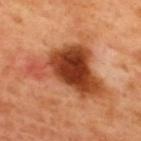Q: Is there a histopathology result?
A: catalogued during a skin exam; not biopsied
Q: Lesion location?
A: the back
Q: Who is the patient?
A: male, roughly 50 years of age
Q: What is the lesion's diameter?
A: ~9.5 mm (longest diameter)
Q: Automated lesion metrics?
A: a footprint of about 31 mm² and an eccentricity of roughly 0.8; a mean CIELAB color near L≈45 a*≈30 b*≈38, roughly 17 lightness units darker than nearby skin, and a normalized border contrast of about 11.5; internal color variation of about 9 on a 0–10 scale and a peripheral color-asymmetry measure near 2.5
Q: Illumination type?
A: cross-polarized illumination
Q: What is the imaging modality?
A: 15 mm crop, total-body photography